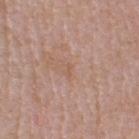Q: Was this lesion biopsied?
A: no biopsy performed (imaged during a skin exam)
Q: Who is the patient?
A: female, in their mid- to late 60s
Q: What kind of image is this?
A: 15 mm crop, total-body photography
Q: Lesion location?
A: the head or neck